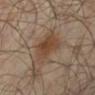follow-up — imaged on a skin check; not biopsied
acquisition — ~15 mm tile from a whole-body skin photo
subject — male, approximately 65 years of age
location — the left lower leg